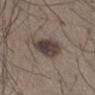biopsy status: total-body-photography surveillance lesion; no biopsy
image-analysis metrics: an area of roughly 12 mm², an outline eccentricity of about 0.55 (0 = round, 1 = elongated), and a symmetry-axis asymmetry near 0.25; an average lesion color of about L≈40 a*≈12 b*≈18 (CIELAB) and a lesion–skin lightness drop of about 12
image: ~15 mm tile from a whole-body skin photo
site: the leg
patient: male, aged around 50
lesion diameter: ~4 mm (longest diameter)